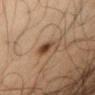The lesion was photographed on a routine skin check and not biopsied; there is no pathology result.
The subject is a male about 50 years old.
The total-body-photography lesion software estimated a mean CIELAB color near L≈41 a*≈16 b*≈28, a lesion–skin lightness drop of about 10, and a lesion-to-skin contrast of about 8.5 (normalized; higher = more distinct). The analysis additionally found a border-irregularity rating of about 4/10, internal color variation of about 4.5 on a 0–10 scale, and a peripheral color-asymmetry measure near 1. The analysis additionally found a classifier nevus-likeness of about 95/100 and a detector confidence of about 100 out of 100 that the crop contains a lesion.
A roughly 15 mm field-of-view crop from a total-body skin photograph.
Imaged with cross-polarized lighting.
Measured at roughly 3.5 mm in maximum diameter.
From the chest.